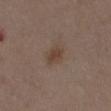Imaged during a routine full-body skin examination; the lesion was not biopsied and no histopathology is available. A region of skin cropped from a whole-body photographic capture, roughly 15 mm wide. The lesion is located on the abdomen. Imaged with white-light lighting. Approximately 3 mm at its widest. Automated image analysis of the tile measured a footprint of about 3.5 mm², a shape eccentricity near 0.75, and a symmetry-axis asymmetry near 0.25. The software also gave an average lesion color of about L≈41 a*≈15 b*≈24 (CIELAB), a lesion–skin lightness drop of about 7, and a lesion-to-skin contrast of about 7 (normalized; higher = more distinct). The software also gave a border-irregularity index near 2/10, a color-variation rating of about 1.5/10, and a peripheral color-asymmetry measure near 0.5. The software also gave a detector confidence of about 100 out of 100 that the crop contains a lesion. A male subject, approximately 50 years of age.